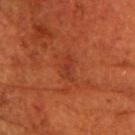Impression: Part of a total-body skin-imaging series; this lesion was reviewed on a skin check and was not flagged for biopsy.